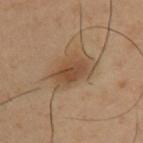Clinical impression:
Captured during whole-body skin photography for melanoma surveillance; the lesion was not biopsied.
Clinical summary:
The lesion's longest dimension is about 4 mm. A male patient, about 40 years old. A 15 mm crop from a total-body photograph taken for skin-cancer surveillance. The lesion is on the upper back. The total-body-photography lesion software estimated a footprint of about 9.5 mm², an outline eccentricity of about 0.75 (0 = round, 1 = elongated), and a symmetry-axis asymmetry near 0.2. The software also gave a mean CIELAB color near L≈47 a*≈18 b*≈32, about 9 CIELAB-L* units darker than the surrounding skin, and a normalized border contrast of about 7.5. The software also gave a border-irregularity index near 2.5/10, a color-variation rating of about 3.5/10, and a peripheral color-asymmetry measure near 1. It also reported a detector confidence of about 100 out of 100 that the crop contains a lesion. This is a cross-polarized tile.